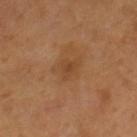biopsy_status: not biopsied; imaged during a skin examination
patient:
  sex: female
  age_approx: 55
image:
  source: total-body photography crop
  field_of_view_mm: 15
site: right lower leg
lesion_size:
  long_diameter_mm_approx: 3.0
lighting: cross-polarized